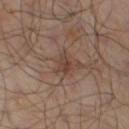{"biopsy_status": "not biopsied; imaged during a skin examination", "image": {"source": "total-body photography crop", "field_of_view_mm": 15}, "site": "left lower leg", "lesion_size": {"long_diameter_mm_approx": 3.0}, "lighting": "cross-polarized", "patient": {"sex": "male", "age_approx": 65}}